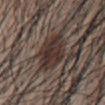| feature | finding |
|---|---|
| biopsy status | total-body-photography surveillance lesion; no biopsy |
| site | the abdomen |
| automated lesion analysis | a lesion–skin lightness drop of about 10 and a normalized lesion–skin contrast near 9.5; a classifier nevus-likeness of about 15/100 and a detector confidence of about 0 out of 100 that the crop contains a lesion |
| subject | male, aged 53–57 |
| lighting | white-light |
| image | ~15 mm tile from a whole-body skin photo |